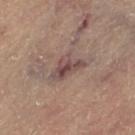Imaged during a routine full-body skin examination; the lesion was not biopsied and no histopathology is available.
Captured under cross-polarized illumination.
The recorded lesion diameter is about 4.5 mm.
The lesion is located on the leg.
A female patient, aged 63 to 67.
This image is a 15 mm lesion crop taken from a total-body photograph.